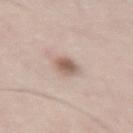Assessment:
No biopsy was performed on this lesion — it was imaged during a full skin examination and was not determined to be concerning.
Image and clinical context:
The patient is a male roughly 65 years of age. The lesion is on the right thigh. Automated tile analysis of the lesion measured a lesion color around L≈59 a*≈16 b*≈24 in CIELAB, a lesion–skin lightness drop of about 13, and a normalized lesion–skin contrast near 8. The tile uses white-light illumination. Approximately 3 mm at its widest. A 15 mm close-up extracted from a 3D total-body photography capture.